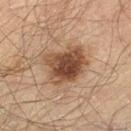Case summary:
- patient — male, approximately 60 years of age
- diameter — ~5 mm (longest diameter)
- body site — the left thigh
- image source — total-body-photography crop, ~15 mm field of view